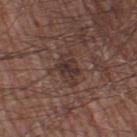Captured during whole-body skin photography for melanoma surveillance; the lesion was not biopsied.
The patient is a male about 60 years old.
The recorded lesion diameter is about 3.5 mm.
A 15 mm close-up tile from a total-body photography series done for melanoma screening.
The lesion is located on the leg.